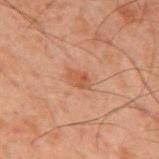Imaged with cross-polarized lighting. The patient is a male aged 58–62. A lesion tile, about 15 mm wide, cut from a 3D total-body photograph. The lesion is on the mid back.